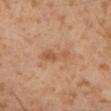The lesion was photographed on a routine skin check and not biopsied; there is no pathology result.
Longest diameter approximately 4 mm.
The patient is a male about 55 years old.
The lesion is on the left lower leg.
This is a cross-polarized tile.
Automated tile analysis of the lesion measured an area of roughly 6 mm², an eccentricity of roughly 0.9, and two-axis asymmetry of about 0.3. The software also gave a classifier nevus-likeness of about 0/100 and a lesion-detection confidence of about 100/100.
This image is a 15 mm lesion crop taken from a total-body photograph.